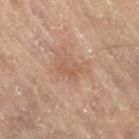Case summary:
- follow-up: imaged on a skin check; not biopsied
- tile lighting: cross-polarized
- image source: ~15 mm tile from a whole-body skin photo
- location: the left leg
- patient: female, aged 78–82